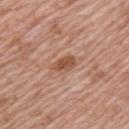Assessment: Captured during whole-body skin photography for melanoma surveillance; the lesion was not biopsied. Clinical summary: Cropped from a total-body skin-imaging series; the visible field is about 15 mm. The lesion is located on the back. A male patient, in their 60s. Captured under white-light illumination. The lesion's longest dimension is about 3 mm. Automated tile analysis of the lesion measured a border-irregularity rating of about 2/10 and a peripheral color-asymmetry measure near 0.5.